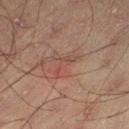<case>
<biopsy_status>not biopsied; imaged during a skin examination</biopsy_status>
<image>
  <source>total-body photography crop</source>
  <field_of_view_mm>15</field_of_view_mm>
</image>
<patient>
  <sex>male</sex>
  <age_approx>50</age_approx>
</patient>
<site>left thigh</site>
</case>